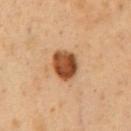{
  "patient": {
    "sex": "male",
    "age_approx": 50
  },
  "image": {
    "source": "total-body photography crop",
    "field_of_view_mm": 15
  },
  "site": "chest",
  "lesion_size": {
    "long_diameter_mm_approx": 3.5
  },
  "automated_metrics": {
    "area_mm2_approx": 9.0,
    "eccentricity": 0.6,
    "nevus_likeness_0_100": 100,
    "lesion_detection_confidence_0_100": 100
  }
}